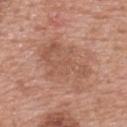Clinical impression: Captured during whole-body skin photography for melanoma surveillance; the lesion was not biopsied. Context: The lesion-visualizer software estimated an automated nevus-likeness rating near 0 out of 100 and lesion-presence confidence of about 100/100. The lesion is located on the upper back. The tile uses white-light illumination. A female subject, aged 58 to 62. A 15 mm crop from a total-body photograph taken for skin-cancer surveillance.